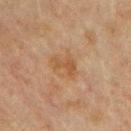workup: imaged on a skin check; not biopsied
illumination: cross-polarized illumination
subject: male, approximately 65 years of age
lesion size: ≈3.5 mm
image source: total-body-photography crop, ~15 mm field of view
location: the upper back
automated metrics: an average lesion color of about L≈42 a*≈17 b*≈30 (CIELAB) and about 6 CIELAB-L* units darker than the surrounding skin; a border-irregularity rating of about 5.5/10, a color-variation rating of about 2/10, and peripheral color asymmetry of about 0.5; a classifier nevus-likeness of about 0/100 and a lesion-detection confidence of about 100/100